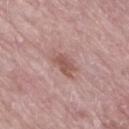The lesion was tiled from a total-body skin photograph and was not biopsied. A female patient about 65 years old. Cropped from a total-body skin-imaging series; the visible field is about 15 mm. On the right thigh. Approximately 3 mm at its widest.